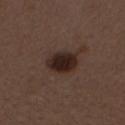Captured during whole-body skin photography for melanoma surveillance; the lesion was not biopsied. Captured under white-light illumination. A region of skin cropped from a whole-body photographic capture, roughly 15 mm wide. On the mid back. Longest diameter approximately 4 mm. The subject is a female roughly 50 years of age.